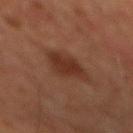This lesion was catalogued during total-body skin photography and was not selected for biopsy. A lesion tile, about 15 mm wide, cut from a 3D total-body photograph. From the chest. A male patient in their 60s.